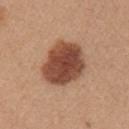<tbp_lesion>
  <biopsy_status>not biopsied; imaged during a skin examination</biopsy_status>
  <patient>
    <sex>female</sex>
    <age_approx>45</age_approx>
  </patient>
  <site>right upper arm</site>
  <image>
    <source>total-body photography crop</source>
    <field_of_view_mm>15</field_of_view_mm>
  </image>
  <lesion_size>
    <long_diameter_mm_approx>6.0</long_diameter_mm_approx>
  </lesion_size>
  <lighting>white-light</lighting>
</tbp_lesion>